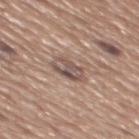Part of a total-body skin-imaging series; this lesion was reviewed on a skin check and was not flagged for biopsy. Cropped from a whole-body photographic skin survey; the tile spans about 15 mm. On the mid back. A male subject aged approximately 65.